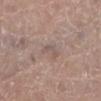- follow-up · imaged on a skin check; not biopsied
- image source · total-body-photography crop, ~15 mm field of view
- image-analysis metrics · an area of roughly 3 mm²; a mean CIELAB color near L≈54 a*≈15 b*≈20 and a lesion-to-skin contrast of about 5 (normalized; higher = more distinct)
- lighting · white-light
- body site · the left lower leg
- patient · female, aged around 60